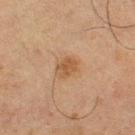workup: total-body-photography surveillance lesion; no biopsy | subject: male, about 65 years old | acquisition: ~15 mm tile from a whole-body skin photo | body site: the right thigh.